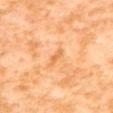Captured during whole-body skin photography for melanoma surveillance; the lesion was not biopsied. A 15 mm crop from a total-body photograph taken for skin-cancer surveillance. A female subject, aged 53 to 57. The lesion's longest dimension is about 2.5 mm. The lesion is on the back. The tile uses cross-polarized illumination.